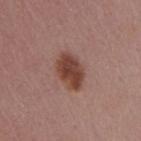From the arm.
Cropped from a total-body skin-imaging series; the visible field is about 15 mm.
A female patient about 45 years old.
Longest diameter approximately 4.5 mm.
Imaged with white-light lighting.
An algorithmic analysis of the crop reported a lesion area of about 10 mm², a shape eccentricity near 0.75, and a symmetry-axis asymmetry near 0.15. And it measured an average lesion color of about L≈43 a*≈22 b*≈26 (CIELAB) and a normalized lesion–skin contrast near 10. The software also gave a border-irregularity rating of about 1.5/10, a within-lesion color-variation index near 3.5/10, and peripheral color asymmetry of about 1. It also reported a classifier nevus-likeness of about 100/100 and a detector confidence of about 100 out of 100 that the crop contains a lesion.